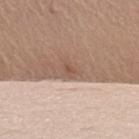The lesion was photographed on a routine skin check and not biopsied; there is no pathology result. A male patient about 55 years old. A close-up tile cropped from a whole-body skin photograph, about 15 mm across. On the upper back.